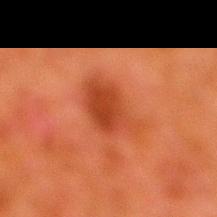workup: imaged on a skin check; not biopsied | site: the right lower leg | image: total-body-photography crop, ~15 mm field of view | subject: male, aged 78 to 82.